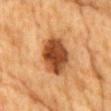Clinical impression: The lesion was tiled from a total-body skin photograph and was not biopsied. Background: A male patient aged approximately 85 or older. Captured under cross-polarized illumination. Automated tile analysis of the lesion measured an outline eccentricity of about 0.75 (0 = round, 1 = elongated) and a symmetry-axis asymmetry near 0.1. Longest diameter approximately 5.5 mm. A 15 mm close-up extracted from a 3D total-body photography capture. Located on the chest.